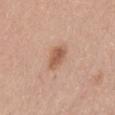About 3 mm across. Located on the abdomen. Captured under white-light illumination. The subject is a female roughly 60 years of age. Cropped from a whole-body photographic skin survey; the tile spans about 15 mm.